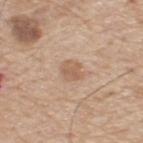The lesion was tiled from a total-body skin photograph and was not biopsied. The lesion is on the upper back. A 15 mm close-up extracted from a 3D total-body photography capture. Longest diameter approximately 2.5 mm. A male patient aged 68 to 72. The total-body-photography lesion software estimated an eccentricity of roughly 0.45 and a shape-asymmetry score of about 0.15 (0 = symmetric). The analysis additionally found a lesion color around L≈59 a*≈18 b*≈31 in CIELAB and a lesion-to-skin contrast of about 6 (normalized; higher = more distinct). The analysis additionally found internal color variation of about 1.5 on a 0–10 scale and radial color variation of about 0.5. The software also gave an automated nevus-likeness rating near 15 out of 100 and lesion-presence confidence of about 100/100.